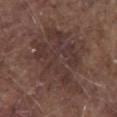Assessment: Recorded during total-body skin imaging; not selected for excision or biopsy. Clinical summary: The recorded lesion diameter is about 10.5 mm. A male patient, aged approximately 80. A lesion tile, about 15 mm wide, cut from a 3D total-body photograph. Imaged with white-light lighting. The lesion is located on the chest.